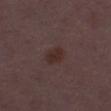The lesion was photographed on a routine skin check and not biopsied; there is no pathology result. A female patient about 30 years old. Captured under white-light illumination. This image is a 15 mm lesion crop taken from a total-body photograph. Automated tile analysis of the lesion measured an area of roughly 4.5 mm². It also reported a mean CIELAB color near L≈27 a*≈16 b*≈18, a lesion–skin lightness drop of about 6, and a lesion-to-skin contrast of about 8 (normalized; higher = more distinct). The analysis additionally found a border-irregularity index near 1.5/10, a color-variation rating of about 2/10, and peripheral color asymmetry of about 1. And it measured a nevus-likeness score of about 70/100. Located on the left thigh. Measured at roughly 3 mm in maximum diameter.